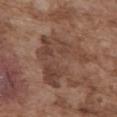No biopsy was performed on this lesion — it was imaged during a full skin examination and was not determined to be concerning.
Located on the abdomen.
A male patient, aged around 75.
A close-up tile cropped from a whole-body skin photograph, about 15 mm across.
This is a white-light tile.
Measured at roughly 7 mm in maximum diameter.